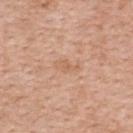The lesion's longest dimension is about 3 mm. This is a white-light tile. A 15 mm close-up tile from a total-body photography series done for melanoma screening. The total-body-photography lesion software estimated a shape eccentricity near 0.9 and a symmetry-axis asymmetry near 0.3. The software also gave a nevus-likeness score of about 0/100. The lesion is located on the back. A female patient aged around 40.